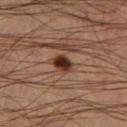Q: Was a biopsy performed?
A: total-body-photography surveillance lesion; no biopsy
Q: Automated lesion metrics?
A: a footprint of about 4 mm², an outline eccentricity of about 0.65 (0 = round, 1 = elongated), and a shape-asymmetry score of about 0.2 (0 = symmetric); a border-irregularity rating of about 2/10, a color-variation rating of about 4.5/10, and a peripheral color-asymmetry measure near 1.5; a lesion-detection confidence of about 100/100
Q: What kind of image is this?
A: ~15 mm tile from a whole-body skin photo
Q: Where on the body is the lesion?
A: the right lower leg
Q: What lighting was used for the tile?
A: cross-polarized
Q: Who is the patient?
A: male, aged 43 to 47